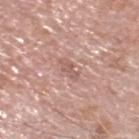Clinical impression: Part of a total-body skin-imaging series; this lesion was reviewed on a skin check and was not flagged for biopsy. Context: The lesion is located on the head or neck. A male subject, aged around 70. Cropped from a total-body skin-imaging series; the visible field is about 15 mm.